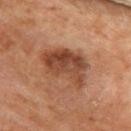– follow-up: no biopsy performed (imaged during a skin exam)
– anatomic site: the upper back
– image: total-body-photography crop, ~15 mm field of view
– illumination: cross-polarized illumination
– size: about 6 mm
– subject: female, roughly 65 years of age